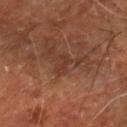{
  "biopsy_status": "not biopsied; imaged during a skin examination",
  "patient": {
    "age_approx": 65
  },
  "lesion_size": {
    "long_diameter_mm_approx": 2.5
  },
  "lighting": "cross-polarized",
  "site": "right lower leg",
  "image": {
    "source": "total-body photography crop",
    "field_of_view_mm": 15
  }
}